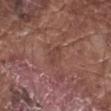Part of a total-body skin-imaging series; this lesion was reviewed on a skin check and was not flagged for biopsy.
Longest diameter approximately 2.5 mm.
A male subject, aged 73 to 77.
A 15 mm close-up extracted from a 3D total-body photography capture.
From the right forearm.
Imaged with white-light lighting.